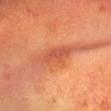Q: Was this lesion biopsied?
A: imaged on a skin check; not biopsied
Q: What did automated image analysis measure?
A: an average lesion color of about L≈51 a*≈30 b*≈35 (CIELAB) and roughly 9 lightness units darker than nearby skin; a border-irregularity rating of about 3/10, internal color variation of about 2 on a 0–10 scale, and a peripheral color-asymmetry measure near 0.5; an automated nevus-likeness rating near 5 out of 100 and a detector confidence of about 75 out of 100 that the crop contains a lesion
Q: Lesion size?
A: about 5 mm
Q: Illumination type?
A: cross-polarized
Q: How was this image acquired?
A: ~15 mm crop, total-body skin-cancer survey
Q: Patient demographics?
A: male, aged approximately 60
Q: Where on the body is the lesion?
A: the head or neck